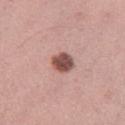Part of a total-body skin-imaging series; this lesion was reviewed on a skin check and was not flagged for biopsy.
Imaged with white-light lighting.
The lesion is on the left thigh.
A region of skin cropped from a whole-body photographic capture, roughly 15 mm wide.
About 3 mm across.
A female patient aged 33–37.